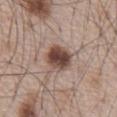Recorded during total-body skin imaging; not selected for excision or biopsy.
The total-body-photography lesion software estimated a lesion area of about 8.5 mm², an outline eccentricity of about 0.45 (0 = round, 1 = elongated), and a shape-asymmetry score of about 0.1 (0 = symmetric). The software also gave a mean CIELAB color near L≈45 a*≈18 b*≈23 and roughly 16 lightness units darker than nearby skin. It also reported border irregularity of about 1.5 on a 0–10 scale and internal color variation of about 5.5 on a 0–10 scale.
A male subject aged around 65.
The recorded lesion diameter is about 3.5 mm.
The lesion is on the chest.
A lesion tile, about 15 mm wide, cut from a 3D total-body photograph.